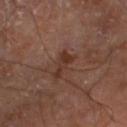{
  "biopsy_status": "not biopsied; imaged during a skin examination",
  "site": "left lower leg",
  "image": {
    "source": "total-body photography crop",
    "field_of_view_mm": 15
  },
  "patient": {
    "sex": "male",
    "age_approx": 70
  },
  "lighting": "cross-polarized",
  "automated_metrics": {
    "cielab_L": 32,
    "cielab_a": 20,
    "cielab_b": 25,
    "vs_skin_contrast_norm": 7.5
  }
}